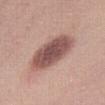Assessment: The lesion was tiled from a total-body skin photograph and was not biopsied. Clinical summary: The subject is a female aged 18–22. Imaged with white-light lighting. Approximately 6.5 mm at its widest. From the right thigh. The lesion-visualizer software estimated a lesion area of about 19 mm² and a shape-asymmetry score of about 0.1 (0 = symmetric). The software also gave an average lesion color of about L≈52 a*≈21 b*≈22 (CIELAB), a lesion–skin lightness drop of about 15, and a lesion-to-skin contrast of about 10.5 (normalized; higher = more distinct). It also reported a border-irregularity index near 1.5/10, a within-lesion color-variation index near 4/10, and radial color variation of about 1. It also reported an automated nevus-likeness rating near 45 out of 100. A roughly 15 mm field-of-view crop from a total-body skin photograph.